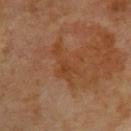Captured during whole-body skin photography for melanoma surveillance; the lesion was not biopsied.
Longest diameter approximately 5.5 mm.
A 15 mm crop from a total-body photograph taken for skin-cancer surveillance.
The lesion is on the upper back.
This is a cross-polarized tile.
The subject is a female aged 68–72.